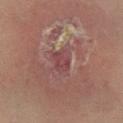notes: catalogued during a skin exam; not biopsied | tile lighting: cross-polarized | anatomic site: the leg | lesion diameter: ≈2.5 mm | patient: female, about 70 years old | image source: ~15 mm crop, total-body skin-cancer survey.